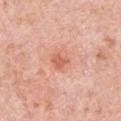notes: no biopsy performed (imaged during a skin exam)
patient: male, roughly 80 years of age
diameter: ≈2.5 mm
automated metrics: an outline eccentricity of about 0.4 (0 = round, 1 = elongated); a border-irregularity index near 1.5/10, a color-variation rating of about 3/10, and radial color variation of about 1
location: the left upper arm
acquisition: 15 mm crop, total-body photography
illumination: white-light